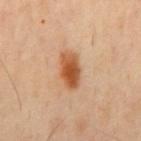| feature | finding |
|---|---|
| follow-up | catalogued during a skin exam; not biopsied |
| acquisition | 15 mm crop, total-body photography |
| size | ~4.5 mm (longest diameter) |
| subject | male, in their mid-40s |
| body site | the back |
| tile lighting | cross-polarized illumination |
| TBP lesion metrics | an outline eccentricity of about 0.85 (0 = round, 1 = elongated) and a symmetry-axis asymmetry near 0.15; a mean CIELAB color near L≈52 a*≈23 b*≈37, roughly 13 lightness units darker than nearby skin, and a normalized border contrast of about 10; border irregularity of about 1.5 on a 0–10 scale, a color-variation rating of about 5.5/10, and a peripheral color-asymmetry measure near 1.5; a classifier nevus-likeness of about 100/100 and a detector confidence of about 100 out of 100 that the crop contains a lesion |